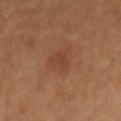<lesion>
  <biopsy_status>not biopsied; imaged during a skin examination</biopsy_status>
  <patient>
    <sex>female</sex>
    <age_approx>40</age_approx>
  </patient>
  <image>
    <source>total-body photography crop</source>
    <field_of_view_mm>15</field_of_view_mm>
  </image>
  <site>left arm</site>
</lesion>